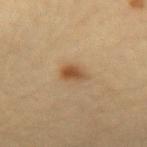Notes:
– notes — total-body-photography surveillance lesion; no biopsy
– lighting — cross-polarized
– patient — female, about 30 years old
– acquisition — total-body-photography crop, ~15 mm field of view
– image-analysis metrics — a lesion–skin lightness drop of about 10 and a normalized border contrast of about 8.5; a border-irregularity index near 3/10 and a peripheral color-asymmetry measure near 1
– anatomic site — the arm
– lesion size — ≈3 mm